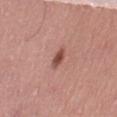– notes: total-body-photography surveillance lesion; no biopsy
– subject: female, about 40 years old
– image source: ~15 mm crop, total-body skin-cancer survey
– anatomic site: the right thigh
– image-analysis metrics: an area of roughly 3 mm², a shape eccentricity near 0.9, and a symmetry-axis asymmetry near 0.3; a border-irregularity index near 3/10 and a within-lesion color-variation index near 2.5/10
– lesion diameter: about 3 mm